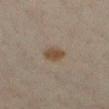Assessment:
Captured during whole-body skin photography for melanoma surveillance; the lesion was not biopsied.
Clinical summary:
A female patient, roughly 45 years of age. The lesion is located on the left lower leg. A lesion tile, about 15 mm wide, cut from a 3D total-body photograph. The tile uses cross-polarized illumination.